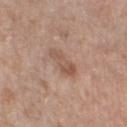No biopsy was performed on this lesion — it was imaged during a full skin examination and was not determined to be concerning.
The lesion is located on the left thigh.
A lesion tile, about 15 mm wide, cut from a 3D total-body photograph.
The patient is a female aged 73 to 77.
This is a white-light tile.